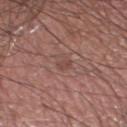Captured during whole-body skin photography for melanoma surveillance; the lesion was not biopsied.
The lesion is on the leg.
A male subject in their mid-40s.
Cropped from a total-body skin-imaging series; the visible field is about 15 mm.